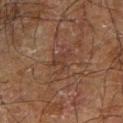notes — catalogued during a skin exam; not biopsied | TBP lesion metrics — a lesion-detection confidence of about 50/100 | subject — male, aged approximately 70 | diameter — about 3.5 mm | image — ~15 mm crop, total-body skin-cancer survey | tile lighting — cross-polarized illumination | location — the right forearm.